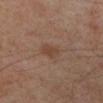workup=imaged on a skin check; not biopsied.